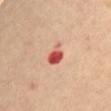notes: total-body-photography surveillance lesion; no biopsy
image-analysis metrics: a footprint of about 4.5 mm², a shape eccentricity near 0.85, and a symmetry-axis asymmetry near 0.35; an average lesion color of about L≈57 a*≈36 b*≈32 (CIELAB), about 17 CIELAB-L* units darker than the surrounding skin, and a lesion-to-skin contrast of about 10.5 (normalized; higher = more distinct); a border-irregularity index near 3.5/10, a color-variation rating of about 5.5/10, and peripheral color asymmetry of about 1.5
patient: female, approximately 60 years of age
imaging modality: ~15 mm tile from a whole-body skin photo
lesion diameter: about 3.5 mm
body site: the abdomen